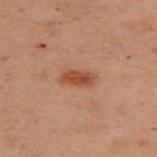| field | value |
|---|---|
| biopsy status | no biopsy performed (imaged during a skin exam) |
| subject | female, roughly 55 years of age |
| lighting | cross-polarized illumination |
| automated lesion analysis | a footprint of about 5 mm², a shape eccentricity near 0.9, and two-axis asymmetry of about 0.2; a lesion color around L≈40 a*≈21 b*≈29 in CIELAB and a normalized lesion–skin contrast near 8; an automated nevus-likeness rating near 85 out of 100 |
| image | ~15 mm crop, total-body skin-cancer survey |
| body site | the upper back |
| diameter | about 3.5 mm |